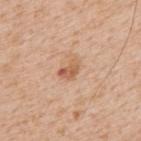Imaged during a routine full-body skin examination; the lesion was not biopsied and no histopathology is available.
The patient is a male about 65 years old.
A close-up tile cropped from a whole-body skin photograph, about 15 mm across.
The lesion is on the upper back.